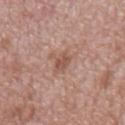Q: Was a biopsy performed?
A: no biopsy performed (imaged during a skin exam)
Q: What kind of image is this?
A: 15 mm crop, total-body photography
Q: What is the lesion's diameter?
A: about 3 mm
Q: Lesion location?
A: the mid back
Q: Illumination type?
A: white-light illumination
Q: Who is the patient?
A: male, in their mid-60s